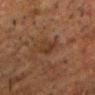Recorded during total-body skin imaging; not selected for excision or biopsy.
Longest diameter approximately 3 mm.
Located on the head or neck.
The patient is a male aged approximately 60.
Cropped from a whole-body photographic skin survey; the tile spans about 15 mm.